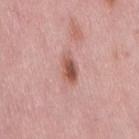  biopsy_status: not biopsied; imaged during a skin examination
  site: back
  patient:
    sex: female
    age_approx: 50
  image:
    source: total-body photography crop
    field_of_view_mm: 15
  lighting: white-light